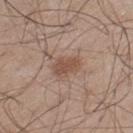Impression: The lesion was tiled from a total-body skin photograph and was not biopsied. Context: A male subject, in their mid-40s. A lesion tile, about 15 mm wide, cut from a 3D total-body photograph. Located on the left thigh. Automated image analysis of the tile measured a footprint of about 5.5 mm², an eccentricity of roughly 0.85, and a shape-asymmetry score of about 0.25 (0 = symmetric). It also reported a lesion color around L≈50 a*≈18 b*≈27 in CIELAB and a lesion-to-skin contrast of about 7 (normalized; higher = more distinct). And it measured an automated nevus-likeness rating near 90 out of 100 and a lesion-detection confidence of about 100/100. The recorded lesion diameter is about 3.5 mm. This is a white-light tile.